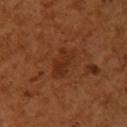Assessment:
The lesion was photographed on a routine skin check and not biopsied; there is no pathology result.
Background:
This image is a 15 mm lesion crop taken from a total-body photograph. The patient is a female aged approximately 55. The lesion is located on the upper back.